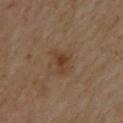Recorded during total-body skin imaging; not selected for excision or biopsy.
The total-body-photography lesion software estimated a lesion area of about 5.5 mm², an eccentricity of roughly 0.75, and a symmetry-axis asymmetry near 0.35. It also reported roughly 8 lightness units darker than nearby skin. The analysis additionally found a nevus-likeness score of about 50/100 and lesion-presence confidence of about 100/100.
About 3.5 mm across.
A male patient, roughly 55 years of age.
The lesion is on the upper back.
A region of skin cropped from a whole-body photographic capture, roughly 15 mm wide.